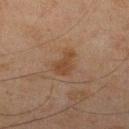Q: Was a biopsy performed?
A: total-body-photography surveillance lesion; no biopsy
Q: How large is the lesion?
A: about 3.5 mm
Q: What lighting was used for the tile?
A: cross-polarized
Q: How was this image acquired?
A: ~15 mm crop, total-body skin-cancer survey
Q: Automated lesion metrics?
A: a border-irregularity rating of about 4/10 and a within-lesion color-variation index near 1.5/10
Q: What are the patient's age and sex?
A: male, about 45 years old
Q: What is the anatomic site?
A: the right lower leg